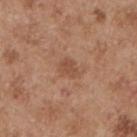Part of a total-body skin-imaging series; this lesion was reviewed on a skin check and was not flagged for biopsy.
The lesion-visualizer software estimated a normalized border contrast of about 5.5. The software also gave a nevus-likeness score of about 15/100.
A male subject about 55 years old.
The lesion is located on the left upper arm.
The recorded lesion diameter is about 2.5 mm.
Cropped from a total-body skin-imaging series; the visible field is about 15 mm.